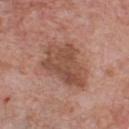notes: catalogued during a skin exam; not biopsied | image: ~15 mm crop, total-body skin-cancer survey | subject: male, approximately 60 years of age | tile lighting: white-light illumination | anatomic site: the chest | diameter: ~6.5 mm (longest diameter).